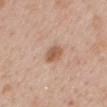An algorithmic analysis of the crop reported a footprint of about 4 mm², a shape eccentricity near 0.8, and a symmetry-axis asymmetry near 0.2. Longest diameter approximately 3 mm. Cropped from a whole-body photographic skin survey; the tile spans about 15 mm. The lesion is on the back. The tile uses white-light illumination. The subject is a female aged 48–52.